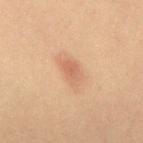Clinical impression: Part of a total-body skin-imaging series; this lesion was reviewed on a skin check and was not flagged for biopsy. Clinical summary: The patient is a male in their 60s. Cropped from a total-body skin-imaging series; the visible field is about 15 mm. From the mid back. An algorithmic analysis of the crop reported a lesion color around L≈51 a*≈19 b*≈29 in CIELAB, a lesion–skin lightness drop of about 7, and a normalized border contrast of about 5.5. And it measured a border-irregularity rating of about 3/10, a within-lesion color-variation index near 2/10, and a peripheral color-asymmetry measure near 0.5. And it measured a classifier nevus-likeness of about 80/100 and a lesion-detection confidence of about 100/100. Measured at roughly 3 mm in maximum diameter.